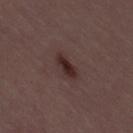biopsy status: total-body-photography surveillance lesion; no biopsy | size: ~3.5 mm (longest diameter) | image source: ~15 mm tile from a whole-body skin photo | subject: female, aged around 30 | body site: the right thigh | illumination: white-light illumination.